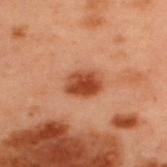biopsy status — imaged on a skin check; not biopsied | subject — male, approximately 55 years of age | lesion diameter — ~3.5 mm (longest diameter) | location — the upper back | image — ~15 mm crop, total-body skin-cancer survey | lighting — cross-polarized.